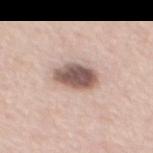Notes:
– biopsy status — imaged on a skin check; not biopsied
– subject — female, aged 43 to 47
– anatomic site — the mid back
– lesion diameter — ~4.5 mm (longest diameter)
– lighting — white-light
– acquisition — 15 mm crop, total-body photography